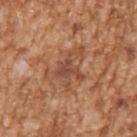Impression: Captured during whole-body skin photography for melanoma surveillance; the lesion was not biopsied. Image and clinical context: A male subject aged 43 to 47. A 15 mm close-up tile from a total-body photography series done for melanoma screening. The lesion is located on the arm.